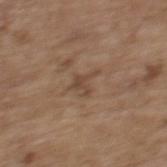Impression:
This lesion was catalogued during total-body skin photography and was not selected for biopsy.
Image and clinical context:
The recorded lesion diameter is about 2.5 mm. A 15 mm close-up extracted from a 3D total-body photography capture. A female subject, in their mid- to late 60s. On the back. Captured under white-light illumination.